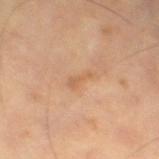Captured during whole-body skin photography for melanoma surveillance; the lesion was not biopsied.
A male subject in their mid- to late 60s.
Located on the left thigh.
A 15 mm close-up tile from a total-body photography series done for melanoma screening.
Automated image analysis of the tile measured an outline eccentricity of about 0.95 (0 = round, 1 = elongated) and a shape-asymmetry score of about 0.5 (0 = symmetric). It also reported an average lesion color of about L≈58 a*≈20 b*≈35 (CIELAB). The analysis additionally found a border-irregularity rating of about 5.5/10, internal color variation of about 0 on a 0–10 scale, and radial color variation of about 0. It also reported a nevus-likeness score of about 0/100.